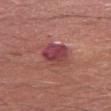Case summary:
• workup · no biopsy performed (imaged during a skin exam)
• site · the right thigh
• tile lighting · white-light illumination
• diameter · about 3.5 mm
• patient · male, in their 60s
• acquisition · total-body-photography crop, ~15 mm field of view